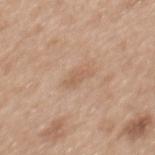{"biopsy_status": "not biopsied; imaged during a skin examination", "lighting": "white-light", "image": {"source": "total-body photography crop", "field_of_view_mm": 15}, "patient": {"sex": "female", "age_approx": 40}, "site": "mid back", "lesion_size": {"long_diameter_mm_approx": 2.5}}